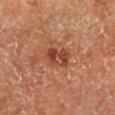{"biopsy_status": "not biopsied; imaged during a skin examination", "patient": {"sex": "male", "age_approx": 65}, "automated_metrics": {"border_irregularity_0_10": 2.0, "color_variation_0_10": 6.5, "peripheral_color_asymmetry": 2.0}, "image": {"source": "total-body photography crop", "field_of_view_mm": 15}, "site": "right lower leg", "lesion_size": {"long_diameter_mm_approx": 4.0}, "lighting": "cross-polarized"}